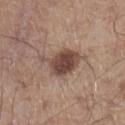Captured during whole-body skin photography for melanoma surveillance; the lesion was not biopsied. About 6 mm across. A close-up tile cropped from a whole-body skin photograph, about 15 mm across. The lesion is located on the left lower leg. A male patient aged around 60. This is a white-light tile.